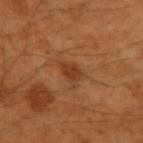lesion_size:
  long_diameter_mm_approx: 2.5
image:
  source: total-body photography crop
  field_of_view_mm: 15
automated_metrics:
  area_mm2_approx: 4.0
  eccentricity: 0.7
  shape_asymmetry: 0.25
  nevus_likeness_0_100: 40
  lesion_detection_confidence_0_100: 100
site: left upper arm
patient:
  sex: male
  age_approx: 50
lighting: cross-polarized